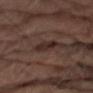notes=no biopsy performed (imaged during a skin exam).